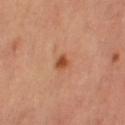{
  "biopsy_status": "not biopsied; imaged during a skin examination",
  "patient": {
    "sex": "female",
    "age_approx": 65
  },
  "lighting": "cross-polarized",
  "lesion_size": {
    "long_diameter_mm_approx": 1.5
  },
  "site": "left thigh",
  "image": {
    "source": "total-body photography crop",
    "field_of_view_mm": 15
  }
}